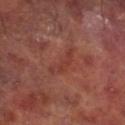Impression:
Captured during whole-body skin photography for melanoma surveillance; the lesion was not biopsied.
Acquisition and patient details:
A roughly 15 mm field-of-view crop from a total-body skin photograph. Captured under cross-polarized illumination. A male patient aged 58 to 62. Measured at roughly 4.5 mm in maximum diameter. The lesion is on the right lower leg.